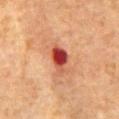Q: Is there a histopathology result?
A: catalogued during a skin exam; not biopsied
Q: Illumination type?
A: cross-polarized illumination
Q: What is the anatomic site?
A: the abdomen
Q: What is the imaging modality?
A: ~15 mm tile from a whole-body skin photo
Q: What is the lesion's diameter?
A: about 4 mm
Q: Who is the patient?
A: male, aged 78–82